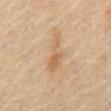Part of a total-body skin-imaging series; this lesion was reviewed on a skin check and was not flagged for biopsy. A male subject in their 70s. Located on the abdomen. A 15 mm close-up extracted from a 3D total-body photography capture. This is a cross-polarized tile. Approximately 5 mm at its widest.